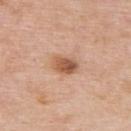{
  "biopsy_status": "not biopsied; imaged during a skin examination"
}